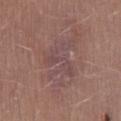Assessment: Part of a total-body skin-imaging series; this lesion was reviewed on a skin check and was not flagged for biopsy. Image and clinical context: The patient is a female approximately 35 years of age. About 7 mm across. Cropped from a total-body skin-imaging series; the visible field is about 15 mm. The lesion is on the lower back. This is a white-light tile.